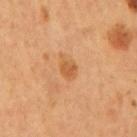| feature | finding |
|---|---|
| subject | male, aged 53 to 57 |
| location | the mid back |
| tile lighting | cross-polarized illumination |
| lesion diameter | ~3 mm (longest diameter) |
| acquisition | 15 mm crop, total-body photography |